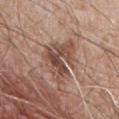Impression: The lesion was photographed on a routine skin check and not biopsied; there is no pathology result. Clinical summary: From the back. An algorithmic analysis of the crop reported a mean CIELAB color near L≈48 a*≈19 b*≈25. It also reported a color-variation rating of about 5.5/10 and a peripheral color-asymmetry measure near 1.5. The software also gave a nevus-likeness score of about 0/100 and lesion-presence confidence of about 95/100. This is a white-light tile. A roughly 15 mm field-of-view crop from a total-body skin photograph. Longest diameter approximately 6 mm. A male subject aged 58 to 62.